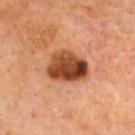Recorded during total-body skin imaging; not selected for excision or biopsy. An algorithmic analysis of the crop reported a footprint of about 14 mm² and a shape-asymmetry score of about 0.2 (0 = symmetric). It also reported border irregularity of about 2 on a 0–10 scale, internal color variation of about 9.5 on a 0–10 scale, and a peripheral color-asymmetry measure near 3.5. The software also gave a classifier nevus-likeness of about 70/100. A lesion tile, about 15 mm wide, cut from a 3D total-body photograph. Imaged with cross-polarized lighting. A male subject, aged 53 to 57. The lesion is on the upper back.